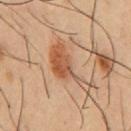workup: total-body-photography surveillance lesion; no biopsy